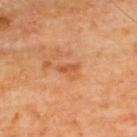workup = no biopsy performed (imaged during a skin exam) | body site = the back | diameter = ≈2.5 mm | image-analysis metrics = an area of roughly 2 mm², an eccentricity of roughly 0.9, and two-axis asymmetry of about 0.4; a lesion-detection confidence of about 100/100 | patient = male, aged approximately 65 | lighting = cross-polarized illumination | image = 15 mm crop, total-body photography.